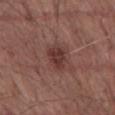The lesion was tiled from a total-body skin photograph and was not biopsied. The lesion's longest dimension is about 3 mm. A roughly 15 mm field-of-view crop from a total-body skin photograph. Imaged with white-light lighting. The lesion is on the abdomen. A male patient aged approximately 55. The total-body-photography lesion software estimated an outline eccentricity of about 0.55 (0 = round, 1 = elongated) and two-axis asymmetry of about 0.25. It also reported a lesion color around L≈36 a*≈22 b*≈22 in CIELAB, a lesion–skin lightness drop of about 9, and a normalized border contrast of about 8. The software also gave a border-irregularity index near 2.5/10 and radial color variation of about 1.